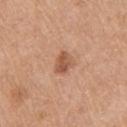Part of a total-body skin-imaging series; this lesion was reviewed on a skin check and was not flagged for biopsy. This is a white-light tile. Located on the upper back. The subject is a male aged around 60. A roughly 15 mm field-of-view crop from a total-body skin photograph.